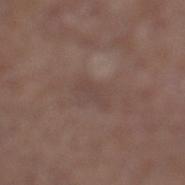body site: the left lower leg; lesion size: ~3 mm (longest diameter); acquisition: 15 mm crop, total-body photography; tile lighting: white-light illumination; TBP lesion metrics: a normalized border contrast of about 4.5; subject: male, aged approximately 55.